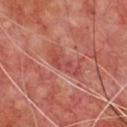lighting — cross-polarized illumination | TBP lesion metrics — a lesion color around L≈47 a*≈32 b*≈27 in CIELAB, a lesion–skin lightness drop of about 7, and a normalized border contrast of about 5.5; a border-irregularity rating of about 5/10 | patient — male, about 70 years old | diameter — ~4.5 mm (longest diameter) | acquisition — 15 mm crop, total-body photography | site — the chest.